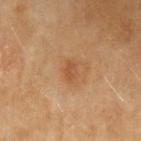  biopsy_status: not biopsied; imaged during a skin examination
  patient:
    sex: female
    age_approx: 60
  image:
    source: total-body photography crop
    field_of_view_mm: 15
  site: left upper arm